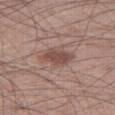Q: Was this lesion biopsied?
A: no biopsy performed (imaged during a skin exam)
Q: Lesion location?
A: the right thigh
Q: What are the patient's age and sex?
A: male, in their mid-40s
Q: How was the tile lit?
A: white-light
Q: What kind of image is this?
A: ~15 mm crop, total-body skin-cancer survey